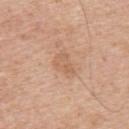notes = no biopsy performed (imaged during a skin exam); patient = male, in their mid-60s; body site = the upper back; imaging modality = ~15 mm crop, total-body skin-cancer survey.